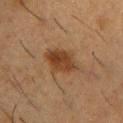| key | value |
|---|---|
| follow-up | total-body-photography surveillance lesion; no biopsy |